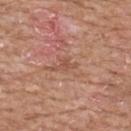On the front of the torso.
About 2.5 mm across.
The patient is a male aged 58 to 62.
The tile uses white-light illumination.
This image is a 15 mm lesion crop taken from a total-body photograph.
Automated tile analysis of the lesion measured border irregularity of about 6 on a 0–10 scale, a within-lesion color-variation index near 0/10, and radial color variation of about 0. The analysis additionally found a lesion-detection confidence of about 95/100.